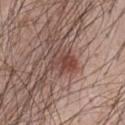<lesion>
  <biopsy_status>not biopsied; imaged during a skin examination</biopsy_status>
  <image>
    <source>total-body photography crop</source>
    <field_of_view_mm>15</field_of_view_mm>
  </image>
  <lesion_size>
    <long_diameter_mm_approx>3.5</long_diameter_mm_approx>
  </lesion_size>
  <lighting>white-light</lighting>
  <site>chest</site>
  <patient>
    <sex>male</sex>
    <age_approx>55</age_approx>
  </patient>
  <automated_metrics>
    <area_mm2_approx>8.0</area_mm2_approx>
    <eccentricity>0.6</eccentricity>
    <shape_asymmetry>0.35</shape_asymmetry>
    <cielab_L>45</cielab_L>
    <cielab_a>20</cielab_a>
    <cielab_b>23</cielab_b>
    <vs_skin_darker_L>9.0</vs_skin_darker_L>
    <border_irregularity_0_10>3.5</border_irregularity_0_10>
    <peripheral_color_asymmetry>2.0</peripheral_color_asymmetry>
    <lesion_detection_confidence_0_100>100</lesion_detection_confidence_0_100>
  </automated_metrics>
</lesion>